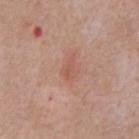notes = imaged on a skin check; not biopsied | patient = male, aged 63–67 | image = ~15 mm crop, total-body skin-cancer survey | site = the abdomen.